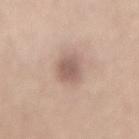The lesion was photographed on a routine skin check and not biopsied; there is no pathology result. The tile uses white-light illumination. Approximately 4 mm at its widest. The subject is a male aged approximately 50. A 15 mm close-up tile from a total-body photography series done for melanoma screening. The lesion is on the lower back. The total-body-photography lesion software estimated about 11 CIELAB-L* units darker than the surrounding skin. The software also gave an automated nevus-likeness rating near 20 out of 100 and a detector confidence of about 100 out of 100 that the crop contains a lesion.